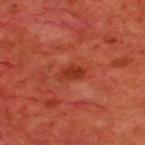This lesion was catalogued during total-body skin photography and was not selected for biopsy.
The lesion-visualizer software estimated a mean CIELAB color near L≈35 a*≈34 b*≈35, roughly 8 lightness units darker than nearby skin, and a lesion-to-skin contrast of about 7.5 (normalized; higher = more distinct).
This image is a 15 mm lesion crop taken from a total-body photograph.
The patient is a male aged 58 to 62.
The lesion is on the upper back.
Imaged with cross-polarized lighting.